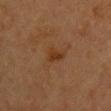Case summary:
* workup · catalogued during a skin exam; not biopsied
* automated lesion analysis · a footprint of about 4 mm², an eccentricity of roughly 0.7, and a symmetry-axis asymmetry near 0.4; an average lesion color of about L≈31 a*≈19 b*≈31 (CIELAB); a border-irregularity rating of about 3.5/10, a color-variation rating of about 2/10, and peripheral color asymmetry of about 0.5
* illumination · cross-polarized
* subject · female, approximately 60 years of age
* imaging modality · 15 mm crop, total-body photography
* lesion diameter · about 2.5 mm
* anatomic site · the back